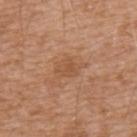Clinical impression:
This lesion was catalogued during total-body skin photography and was not selected for biopsy.
Background:
A roughly 15 mm field-of-view crop from a total-body skin photograph. Captured under white-light illumination. The total-body-photography lesion software estimated a footprint of about 5.5 mm², an outline eccentricity of about 0.75 (0 = round, 1 = elongated), and two-axis asymmetry of about 0.35. The software also gave a mean CIELAB color near L≈52 a*≈22 b*≈34, roughly 7 lightness units darker than nearby skin, and a normalized lesion–skin contrast near 5.5. It also reported a color-variation rating of about 2/10 and peripheral color asymmetry of about 0.5. Measured at roughly 3.5 mm in maximum diameter. A male subject, approximately 75 years of age. The lesion is located on the upper back.